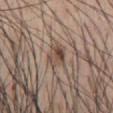biopsy_status: not biopsied; imaged during a skin examination
patient:
  sex: male
  age_approx: 55
image:
  source: total-body photography crop
  field_of_view_mm: 15
site: abdomen
lesion_size:
  long_diameter_mm_approx: 3.0